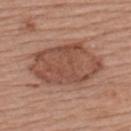Case summary:
* follow-up: imaged on a skin check; not biopsied
* subject: female, about 55 years old
* acquisition: ~15 mm crop, total-body skin-cancer survey
* anatomic site: the back